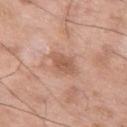The lesion was tiled from a total-body skin photograph and was not biopsied.
The lesion's longest dimension is about 3 mm.
This image is a 15 mm lesion crop taken from a total-body photograph.
The lesion is located on the left thigh.
A male subject aged 48 to 52.